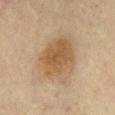location = the front of the torso
lesion diameter = ≈6 mm
patient = male, about 65 years old
tile lighting = cross-polarized
imaging modality = ~15 mm tile from a whole-body skin photo